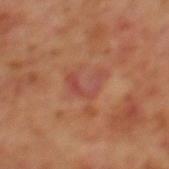notes: total-body-photography surveillance lesion; no biopsy
acquisition: ~15 mm tile from a whole-body skin photo
TBP lesion metrics: a border-irregularity rating of about 9/10, a color-variation rating of about 1/10, and a peripheral color-asymmetry measure near 0
tile lighting: cross-polarized illumination
lesion size: ≈4 mm
site: the mid back
subject: male, approximately 70 years of age